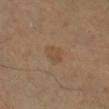  biopsy_status: not biopsied; imaged during a skin examination
  site: right lower leg
  lesion_size:
    long_diameter_mm_approx: 2.5
  patient:
    sex: male
    age_approx: 45
  image:
    source: total-body photography crop
    field_of_view_mm: 15
  lighting: cross-polarized
  automated_metrics:
    area_mm2_approx: 4.0
    eccentricity: 0.75
    shape_asymmetry: 0.3
    cielab_L: 46
    cielab_a: 16
    cielab_b: 31
    vs_skin_darker_L: 5.0
    vs_skin_contrast_norm: 5.0
    border_irregularity_0_10: 3.0